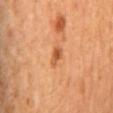Clinical impression:
Recorded during total-body skin imaging; not selected for excision or biopsy.
Context:
The lesion's longest dimension is about 2.5 mm. This is a cross-polarized tile. The patient is female. The lesion is located on the back. Cropped from a total-body skin-imaging series; the visible field is about 15 mm.